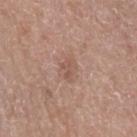Assessment: The lesion was tiled from a total-body skin photograph and was not biopsied. Clinical summary: Measured at roughly 2.5 mm in maximum diameter. A lesion tile, about 15 mm wide, cut from a 3D total-body photograph. The tile uses white-light illumination. A female patient, aged around 50. The lesion is on the left upper arm.